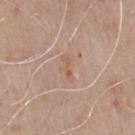Context:
A region of skin cropped from a whole-body photographic capture, roughly 15 mm wide. On the chest. Captured under white-light illumination. The lesion's longest dimension is about 3 mm. A male subject approximately 75 years of age. The lesion-visualizer software estimated a footprint of about 4 mm² and a shape-asymmetry score of about 0.35 (0 = symmetric). The software also gave about 6 CIELAB-L* units darker than the surrounding skin and a lesion-to-skin contrast of about 5.5 (normalized; higher = more distinct).